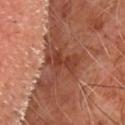notes=catalogued during a skin exam; not biopsied | size=~4 mm (longest diameter) | patient=male, aged around 65 | image=~15 mm tile from a whole-body skin photo | image-analysis metrics=a mean CIELAB color near L≈39 a*≈26 b*≈30, roughly 9 lightness units darker than nearby skin, and a normalized border contrast of about 7.5; a nevus-likeness score of about 0/100 and lesion-presence confidence of about 85/100 | illumination=cross-polarized.